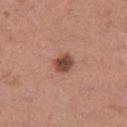follow-up: catalogued during a skin exam; not biopsied | size: ~2.5 mm (longest diameter) | imaging modality: 15 mm crop, total-body photography | TBP lesion metrics: a mean CIELAB color near L≈47 a*≈24 b*≈28; border irregularity of about 1.5 on a 0–10 scale, internal color variation of about 3 on a 0–10 scale, and peripheral color asymmetry of about 1 | patient: female, aged 28 to 32 | anatomic site: the right thigh.